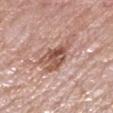Part of a total-body skin-imaging series; this lesion was reviewed on a skin check and was not flagged for biopsy. Automated image analysis of the tile measured a lesion area of about 11 mm², an eccentricity of roughly 0.9, and two-axis asymmetry of about 0.4. And it measured a lesion color around L≈54 a*≈22 b*≈27 in CIELAB, a lesion–skin lightness drop of about 12, and a lesion-to-skin contrast of about 8.5 (normalized; higher = more distinct). The software also gave border irregularity of about 5.5 on a 0–10 scale, a color-variation rating of about 7/10, and a peripheral color-asymmetry measure near 2.5. It also reported a nevus-likeness score of about 5/100 and a lesion-detection confidence of about 100/100. A female subject aged 68–72. Imaged with white-light lighting. The lesion is located on the left lower leg. A 15 mm close-up tile from a total-body photography series done for melanoma screening. Measured at roughly 6.5 mm in maximum diameter.